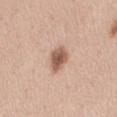{"biopsy_status": "not biopsied; imaged during a skin examination", "lighting": "white-light", "patient": {"sex": "female", "age_approx": 40}, "image": {"source": "total-body photography crop", "field_of_view_mm": 15}, "site": "lower back", "lesion_size": {"long_diameter_mm_approx": 3.5}}